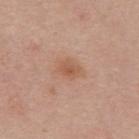The lesion was tiled from a total-body skin photograph and was not biopsied. The tile uses white-light illumination. On the upper back. A roughly 15 mm field-of-view crop from a total-body skin photograph. The subject is a female aged approximately 40. Approximately 3 mm at its widest.